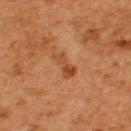Captured during whole-body skin photography for melanoma surveillance; the lesion was not biopsied. A close-up tile cropped from a whole-body skin photograph, about 15 mm across. The patient is a male aged 63 to 67. Automated image analysis of the tile measured a lesion area of about 4 mm², a shape eccentricity near 0.95, and a symmetry-axis asymmetry near 0.4. The software also gave a border-irregularity index near 5/10, a within-lesion color-variation index near 1/10, and radial color variation of about 0. It also reported an automated nevus-likeness rating near 0 out of 100 and lesion-presence confidence of about 100/100. From the upper back.